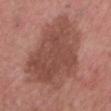Case summary:
• body site — the chest
• image — ~15 mm tile from a whole-body skin photo
• patient — male, in their 50s